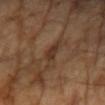Assessment: This lesion was catalogued during total-body skin photography and was not selected for biopsy. Image and clinical context: This is a cross-polarized tile. The subject is a female aged approximately 70. The lesion is on the right forearm. Measured at roughly 3 mm in maximum diameter. This image is a 15 mm lesion crop taken from a total-body photograph.